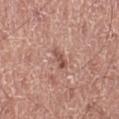This lesion was catalogued during total-body skin photography and was not selected for biopsy. The patient is a male about 60 years old. A lesion tile, about 15 mm wide, cut from a 3D total-body photograph. The lesion-visualizer software estimated a lesion-to-skin contrast of about 7 (normalized; higher = more distinct). And it measured a detector confidence of about 100 out of 100 that the crop contains a lesion. The recorded lesion diameter is about 3 mm. Imaged with white-light lighting. On the leg.